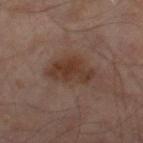biopsy status: catalogued during a skin exam; not biopsied | image source: ~15 mm tile from a whole-body skin photo | tile lighting: cross-polarized | body site: the right thigh | size: about 5.5 mm | patient: male, aged 63–67.